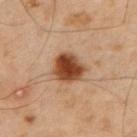Impression:
Recorded during total-body skin imaging; not selected for excision or biopsy.
Image and clinical context:
Measured at roughly 4 mm in maximum diameter. On the back. Cropped from a whole-body photographic skin survey; the tile spans about 15 mm. Captured under cross-polarized illumination. The patient is a male in their 40s.